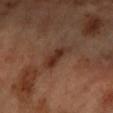Captured during whole-body skin photography for melanoma surveillance; the lesion was not biopsied. From the arm. A female patient about 60 years old. The lesion-visualizer software estimated a footprint of about 6 mm², an outline eccentricity of about 0.9 (0 = round, 1 = elongated), and two-axis asymmetry of about 0.3. It also reported a lesion color around L≈33 a*≈20 b*≈27 in CIELAB, about 9 CIELAB-L* units darker than the surrounding skin, and a normalized border contrast of about 8. And it measured border irregularity of about 3 on a 0–10 scale, a within-lesion color-variation index near 4.5/10, and peripheral color asymmetry of about 1.5. Cropped from a total-body skin-imaging series; the visible field is about 15 mm.